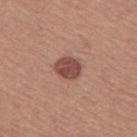The lesion was photographed on a routine skin check and not biopsied; there is no pathology result.
On the left thigh.
A female subject, aged approximately 55.
The lesion's longest dimension is about 3 mm.
A 15 mm crop from a total-body photograph taken for skin-cancer surveillance.